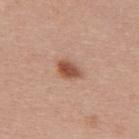Clinical impression:
This lesion was catalogued during total-body skin photography and was not selected for biopsy.